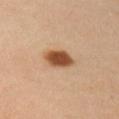Cropped from a total-body skin-imaging series; the visible field is about 15 mm. A male subject, about 30 years old. The tile uses cross-polarized illumination. Measured at roughly 3.5 mm in maximum diameter. On the right upper arm. The total-body-photography lesion software estimated a footprint of about 7 mm² and two-axis asymmetry of about 0.1. The software also gave a border-irregularity rating of about 1/10 and a within-lesion color-variation index near 2.5/10. The analysis additionally found a classifier nevus-likeness of about 100/100 and a lesion-detection confidence of about 100/100.